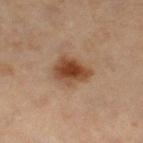Q: Was a biopsy performed?
A: catalogued during a skin exam; not biopsied
Q: Illumination type?
A: cross-polarized illumination
Q: Automated lesion metrics?
A: an area of roughly 10 mm² and a shape-asymmetry score of about 0.2 (0 = symmetric); a lesion color around L≈44 a*≈21 b*≈32 in CIELAB, about 13 CIELAB-L* units darker than the surrounding skin, and a normalized border contrast of about 10.5; a classifier nevus-likeness of about 95/100 and lesion-presence confidence of about 100/100
Q: Patient demographics?
A: female, aged 48 to 52
Q: What is the imaging modality?
A: 15 mm crop, total-body photography
Q: Where on the body is the lesion?
A: the right thigh